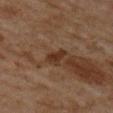  biopsy_status: not biopsied; imaged during a skin examination
  site: upper back
  patient:
    sex: female
    age_approx: 60
  image:
    source: total-body photography crop
    field_of_view_mm: 15